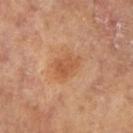The lesion-visualizer software estimated a mean CIELAB color near L≈52 a*≈24 b*≈35, about 8 CIELAB-L* units darker than the surrounding skin, and a normalized lesion–skin contrast near 6. A 15 mm crop from a total-body photograph taken for skin-cancer surveillance. On the left arm. This is a cross-polarized tile. The subject is a female approximately 65 years of age.